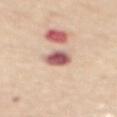workup = imaged on a skin check; not biopsied
imaging modality = 15 mm crop, total-body photography
lighting = white-light illumination
patient = female, aged 63–67
TBP lesion metrics = a border-irregularity rating of about 1.5/10, internal color variation of about 6 on a 0–10 scale, and a peripheral color-asymmetry measure near 2
size = ≈3.5 mm
body site = the mid back